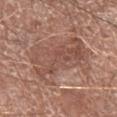follow-up = no biopsy performed (imaged during a skin exam) | patient = male, aged around 80 | body site = the leg | imaging modality = ~15 mm crop, total-body skin-cancer survey | size = ~7.5 mm (longest diameter) | illumination = white-light.